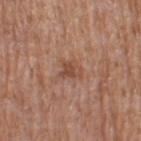Q: What is the anatomic site?
A: the left thigh
Q: How was this image acquired?
A: ~15 mm tile from a whole-body skin photo
Q: What lighting was used for the tile?
A: white-light illumination
Q: Patient demographics?
A: female, in their mid-60s